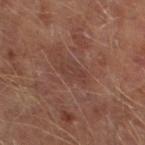notes: no biopsy performed (imaged during a skin exam) | automated lesion analysis: an average lesion color of about L≈38 a*≈20 b*≈23 (CIELAB) and a lesion-to-skin contrast of about 4.5 (normalized; higher = more distinct); a border-irregularity index near 4/10, a within-lesion color-variation index near 2/10, and radial color variation of about 0.5 | diameter: ≈3.5 mm | body site: the left lower leg | patient: male, in their mid-60s | image: 15 mm crop, total-body photography.